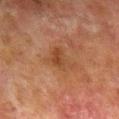biopsy status = catalogued during a skin exam; not biopsied | lighting = cross-polarized illumination | location = the right lower leg | image = total-body-photography crop, ~15 mm field of view | TBP lesion metrics = roughly 6 lightness units darker than nearby skin and a normalized border contrast of about 6; a nevus-likeness score of about 0/100 | patient = male, about 80 years old.